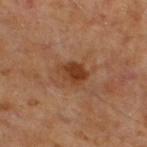Impression:
This lesion was catalogued during total-body skin photography and was not selected for biopsy.
Acquisition and patient details:
A male subject aged 58 to 62. An algorithmic analysis of the crop reported a lesion color around L≈36 a*≈21 b*≈32 in CIELAB, a lesion–skin lightness drop of about 9, and a normalized border contrast of about 9. It also reported border irregularity of about 2 on a 0–10 scale and a peripheral color-asymmetry measure near 1.5. It also reported a classifier nevus-likeness of about 80/100 and a lesion-detection confidence of about 100/100. The lesion is located on the left lower leg. A 15 mm close-up tile from a total-body photography series done for melanoma screening. The recorded lesion diameter is about 3.5 mm. Imaged with cross-polarized lighting.